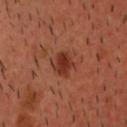Q: Is there a histopathology result?
A: catalogued during a skin exam; not biopsied
Q: Where on the body is the lesion?
A: the head or neck
Q: How was the tile lit?
A: cross-polarized
Q: What did automated image analysis measure?
A: a footprint of about 7 mm², an eccentricity of roughly 0.6, and a symmetry-axis asymmetry near 0.2; a lesion–skin lightness drop of about 10 and a lesion-to-skin contrast of about 8.5 (normalized; higher = more distinct); a border-irregularity rating of about 2/10, internal color variation of about 4 on a 0–10 scale, and a peripheral color-asymmetry measure near 1.5
Q: Lesion size?
A: ~3.5 mm (longest diameter)
Q: Who is the patient?
A: male, aged 38 to 42
Q: What is the imaging modality?
A: total-body-photography crop, ~15 mm field of view